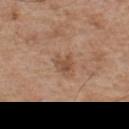Case summary:
– patient: male, aged approximately 55
– image source: 15 mm crop, total-body photography
– location: the front of the torso